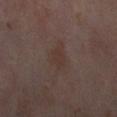The lesion was photographed on a routine skin check and not biopsied; there is no pathology result. The tile uses cross-polarized illumination. A female patient, aged 53 to 57. From the right lower leg. A close-up tile cropped from a whole-body skin photograph, about 15 mm across.